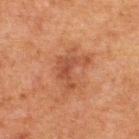Impression:
Part of a total-body skin-imaging series; this lesion was reviewed on a skin check and was not flagged for biopsy.
Context:
Located on the upper back. A male patient, roughly 60 years of age. Longest diameter approximately 4 mm. The tile uses cross-polarized illumination. A 15 mm crop from a total-body photograph taken for skin-cancer surveillance.